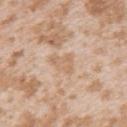No biopsy was performed on this lesion — it was imaged during a full skin examination and was not determined to be concerning. Cropped from a total-body skin-imaging series; the visible field is about 15 mm. The lesion's longest dimension is about 3.5 mm. This is a white-light tile. The lesion is on the left upper arm. A female subject, in their mid- to late 20s.